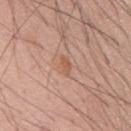<lesion>
<biopsy_status>not biopsied; imaged during a skin examination</biopsy_status>
<site>mid back</site>
<image>
  <source>total-body photography crop</source>
  <field_of_view_mm>15</field_of_view_mm>
</image>
<patient>
  <sex>male</sex>
  <age_approx>60</age_approx>
</patient>
</lesion>